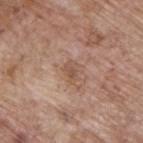A male subject, about 70 years old. On the upper back. Cropped from a total-body skin-imaging series; the visible field is about 15 mm.